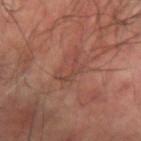Clinical impression: The lesion was tiled from a total-body skin photograph and was not biopsied. Acquisition and patient details: On the left forearm. The tile uses cross-polarized illumination. The subject is a male aged 58–62. Cropped from a whole-body photographic skin survey; the tile spans about 15 mm.